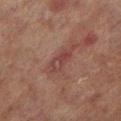The lesion was tiled from a total-body skin photograph and was not biopsied. Located on the left lower leg. A 15 mm crop from a total-body photograph taken for skin-cancer surveillance. The tile uses cross-polarized illumination. Longest diameter approximately 4.5 mm. A male patient, aged 68–72.